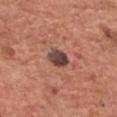Impression: No biopsy was performed on this lesion — it was imaged during a full skin examination and was not determined to be concerning. Context: A male subject, aged around 70. The lesion-visualizer software estimated a footprint of about 5.5 mm² and an outline eccentricity of about 0.65 (0 = round, 1 = elongated). And it measured an average lesion color of about L≈43 a*≈21 b*≈22 (CIELAB), about 15 CIELAB-L* units darker than the surrounding skin, and a normalized border contrast of about 11.5. Approximately 3 mm at its widest. Imaged with white-light lighting. From the head or neck. A close-up tile cropped from a whole-body skin photograph, about 15 mm across.